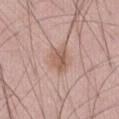Background: Cropped from a whole-body photographic skin survey; the tile spans about 15 mm. The lesion is on the abdomen. Captured under white-light illumination. A male patient approximately 60 years of age. The lesion-visualizer software estimated an area of roughly 5 mm², an outline eccentricity of about 0.7 (0 = round, 1 = elongated), and a symmetry-axis asymmetry near 0.3. The software also gave a mean CIELAB color near L≈57 a*≈19 b*≈27 and a lesion-to-skin contrast of about 7 (normalized; higher = more distinct). And it measured border irregularity of about 3 on a 0–10 scale and radial color variation of about 1.